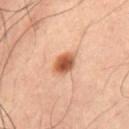  patient:
    sex: male
    age_approx: 60
  site: abdomen
  image:
    source: total-body photography crop
    field_of_view_mm: 15